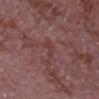Q: Was this lesion biopsied?
A: no biopsy performed (imaged during a skin exam)
Q: What did automated image analysis measure?
A: a lesion color around L≈39 a*≈23 b*≈21 in CIELAB and a lesion–skin lightness drop of about 5
Q: Illumination type?
A: white-light illumination
Q: Lesion size?
A: ≈3 mm
Q: Patient demographics?
A: male, aged 63–67
Q: How was this image acquired?
A: 15 mm crop, total-body photography
Q: Lesion location?
A: the front of the torso